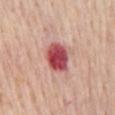workup: catalogued during a skin exam; not biopsied
lighting: white-light
acquisition: ~15 mm tile from a whole-body skin photo
body site: the mid back
subject: male, aged 58 to 62